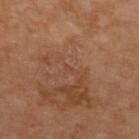| key | value |
|---|---|
| biopsy status | catalogued during a skin exam; not biopsied |
| lighting | cross-polarized illumination |
| diameter | ≈1 mm |
| TBP lesion metrics | a lesion color around L≈45 a*≈23 b*≈29 in CIELAB and a normalized lesion–skin contrast near 3.5 |
| patient | female, aged approximately 60 |
| location | the upper back |
| image source | ~15 mm crop, total-body skin-cancer survey |